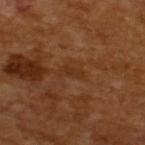No biopsy was performed on this lesion — it was imaged during a full skin examination and was not determined to be concerning.
The patient is a male in their mid-60s.
The lesion's longest dimension is about 3 mm.
Cropped from a total-body skin-imaging series; the visible field is about 15 mm.